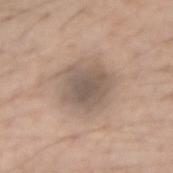Clinical impression: Part of a total-body skin-imaging series; this lesion was reviewed on a skin check and was not flagged for biopsy. Image and clinical context: The total-body-photography lesion software estimated a lesion area of about 17 mm², an eccentricity of roughly 0.4, and a symmetry-axis asymmetry near 0.15. The software also gave an automated nevus-likeness rating near 15 out of 100 and a detector confidence of about 95 out of 100 that the crop contains a lesion. The subject is a male in their mid- to late 60s. The lesion is on the right forearm. A 15 mm close-up tile from a total-body photography series done for melanoma screening. The tile uses white-light illumination.